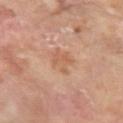Imaged during a routine full-body skin examination; the lesion was not biopsied and no histopathology is available. From the left forearm. The tile uses cross-polarized illumination. About 3.5 mm across. The subject is a female aged 58 to 62. This image is a 15 mm lesion crop taken from a total-body photograph.